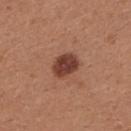The lesion is on the upper back. The lesion's longest dimension is about 3.5 mm. A female patient, aged around 25. Cropped from a whole-body photographic skin survey; the tile spans about 15 mm. Automated image analysis of the tile measured a lesion area of about 7.5 mm², an outline eccentricity of about 0.65 (0 = round, 1 = elongated), and a symmetry-axis asymmetry near 0.15.